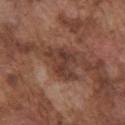Part of a total-body skin-imaging series; this lesion was reviewed on a skin check and was not flagged for biopsy.